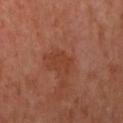notes = catalogued during a skin exam; not biopsied | anatomic site = the right upper arm | tile lighting = cross-polarized illumination | patient = female, aged 48–52 | size = about 3 mm | image source = 15 mm crop, total-body photography | image-analysis metrics = a footprint of about 4.5 mm², a shape eccentricity near 0.75, and two-axis asymmetry of about 0.45; a lesion color around L≈41 a*≈27 b*≈33 in CIELAB and roughly 5 lightness units darker than nearby skin; a within-lesion color-variation index near 0.5/10 and radial color variation of about 0; a classifier nevus-likeness of about 0/100 and lesion-presence confidence of about 100/100.